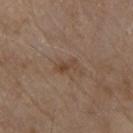Clinical impression:
Captured during whole-body skin photography for melanoma surveillance; the lesion was not biopsied.